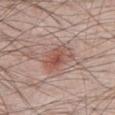Impression: The lesion was tiled from a total-body skin photograph and was not biopsied. Image and clinical context: Measured at roughly 4 mm in maximum diameter. A male subject aged around 40. This is a white-light tile. A 15 mm crop from a total-body photograph taken for skin-cancer surveillance. The lesion is located on the chest.